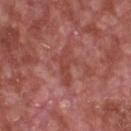No biopsy was performed on this lesion — it was imaged during a full skin examination and was not determined to be concerning. Automated tile analysis of the lesion measured a footprint of about 6 mm² and a shape eccentricity near 0.9. The software also gave an average lesion color of about L≈45 a*≈29 b*≈27 (CIELAB), about 7 CIELAB-L* units darker than the surrounding skin, and a normalized border contrast of about 5.5. And it measured a nevus-likeness score of about 0/100 and lesion-presence confidence of about 70/100. Captured under white-light illumination. The recorded lesion diameter is about 4 mm. A male patient aged 63–67. On the chest. This image is a 15 mm lesion crop taken from a total-body photograph.